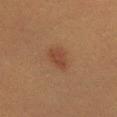Q: Was a biopsy performed?
A: no biopsy performed (imaged during a skin exam)
Q: How was this image acquired?
A: ~15 mm tile from a whole-body skin photo
Q: Automated lesion metrics?
A: about 6 CIELAB-L* units darker than the surrounding skin and a normalized lesion–skin contrast near 6; a peripheral color-asymmetry measure near 0.5
Q: Who is the patient?
A: female, aged around 55
Q: What is the lesion's diameter?
A: about 3.5 mm
Q: What is the anatomic site?
A: the chest